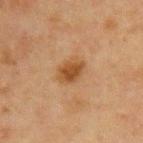Part of a total-body skin-imaging series; this lesion was reviewed on a skin check and was not flagged for biopsy. Measured at roughly 3 mm in maximum diameter. From the chest. This is a cross-polarized tile. The total-body-photography lesion software estimated a lesion area of about 6 mm² and two-axis asymmetry of about 0.2. The software also gave a mean CIELAB color near L≈39 a*≈18 b*≈33 and roughly 9 lightness units darker than nearby skin. The analysis additionally found a border-irregularity rating of about 2/10, a color-variation rating of about 2.5/10, and peripheral color asymmetry of about 1. It also reported an automated nevus-likeness rating near 80 out of 100 and lesion-presence confidence of about 100/100. The subject is a male approximately 65 years of age. A 15 mm close-up extracted from a 3D total-body photography capture.